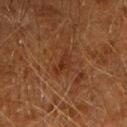The lesion was photographed on a routine skin check and not biopsied; there is no pathology result.
Captured under cross-polarized illumination.
Approximately 3 mm at its widest.
A male patient, roughly 60 years of age.
The lesion is on the arm.
A roughly 15 mm field-of-view crop from a total-body skin photograph.
Automated image analysis of the tile measured a footprint of about 3.5 mm² and an outline eccentricity of about 0.85 (0 = round, 1 = elongated). And it measured a mean CIELAB color near L≈24 a*≈19 b*≈25, about 5 CIELAB-L* units darker than the surrounding skin, and a lesion-to-skin contrast of about 6 (normalized; higher = more distinct). It also reported internal color variation of about 1 on a 0–10 scale and peripheral color asymmetry of about 0.5. The software also gave a classifier nevus-likeness of about 0/100 and a detector confidence of about 95 out of 100 that the crop contains a lesion.